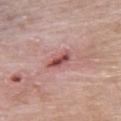Q: Was this lesion biopsied?
A: catalogued during a skin exam; not biopsied
Q: Lesion location?
A: the upper back
Q: Lesion size?
A: ~4 mm (longest diameter)
Q: What are the patient's age and sex?
A: male, in their mid-80s or older
Q: Illumination type?
A: white-light illumination
Q: What kind of image is this?
A: 15 mm crop, total-body photography